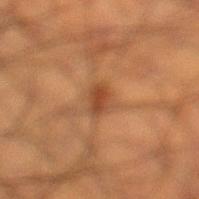Part of a total-body skin-imaging series; this lesion was reviewed on a skin check and was not flagged for biopsy.
On the left lower leg.
Imaged with cross-polarized lighting.
A male patient aged around 50.
The recorded lesion diameter is about 2.5 mm.
A roughly 15 mm field-of-view crop from a total-body skin photograph.